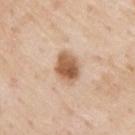The lesion was tiled from a total-body skin photograph and was not biopsied.
A 15 mm close-up extracted from a 3D total-body photography capture.
The lesion is on the upper back.
A male subject, about 80 years old.
Imaged with white-light lighting.
About 3.5 mm across.